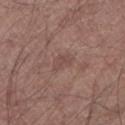No biopsy was performed on this lesion — it was imaged during a full skin examination and was not determined to be concerning. The lesion is located on the left lower leg. The patient is a male aged 53–57. A 15 mm close-up extracted from a 3D total-body photography capture. Longest diameter approximately 2.5 mm. Imaged with white-light lighting.